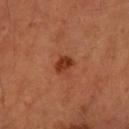| key | value |
|---|---|
| biopsy status | catalogued during a skin exam; not biopsied |
| image | ~15 mm tile from a whole-body skin photo |
| subject | female, aged approximately 60 |
| anatomic site | the left forearm |